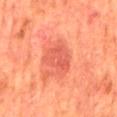Q: What lighting was used for the tile?
A: cross-polarized illumination
Q: Lesion size?
A: ≈4 mm
Q: How was this image acquired?
A: ~15 mm tile from a whole-body skin photo
Q: Where on the body is the lesion?
A: the mid back
Q: Patient demographics?
A: male, in their mid-60s
Q: Automated lesion metrics?
A: a lesion-detection confidence of about 100/100